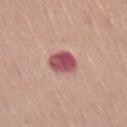size — ~3.5 mm (longest diameter)
location — the abdomen
patient — female, approximately 55 years of age
image-analysis metrics — an average lesion color of about L≈53 a*≈29 b*≈21 (CIELAB), roughly 16 lightness units darker than nearby skin, and a lesion-to-skin contrast of about 11 (normalized; higher = more distinct)
illumination — white-light
image — ~15 mm tile from a whole-body skin photo